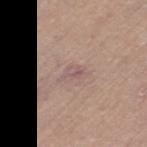Captured during whole-body skin photography for melanoma surveillance; the lesion was not biopsied. An algorithmic analysis of the crop reported a lesion–skin lightness drop of about 6. From the left thigh. The recorded lesion diameter is about 2.5 mm. The tile uses white-light illumination. Cropped from a total-body skin-imaging series; the visible field is about 15 mm. A female subject, aged 53 to 57.